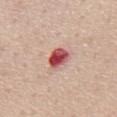follow-up — total-body-photography surveillance lesion; no biopsy.